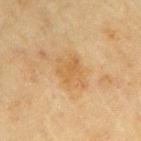Q: Is there a histopathology result?
A: imaged on a skin check; not biopsied
Q: What lighting was used for the tile?
A: cross-polarized
Q: Where on the body is the lesion?
A: the right upper arm
Q: How was this image acquired?
A: total-body-photography crop, ~15 mm field of view
Q: What are the patient's age and sex?
A: male, aged around 70
Q: What did automated image analysis measure?
A: an area of roughly 7.5 mm² and a shape-asymmetry score of about 0.25 (0 = symmetric); a normalized border contrast of about 5.5
Q: Lesion size?
A: ~4 mm (longest diameter)